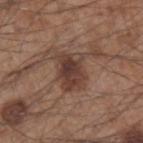Captured during whole-body skin photography for melanoma surveillance; the lesion was not biopsied. Captured under white-light illumination. The lesion is located on the arm. Measured at roughly 4.5 mm in maximum diameter. A male subject aged around 55. A region of skin cropped from a whole-body photographic capture, roughly 15 mm wide.